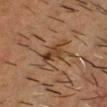biopsy status: imaged on a skin check; not biopsied | image source: ~15 mm crop, total-body skin-cancer survey | diameter: about 3 mm | site: the head or neck | image-analysis metrics: a lesion color around L≈32 a*≈16 b*≈27 in CIELAB, a lesion–skin lightness drop of about 8, and a lesion-to-skin contrast of about 8 (normalized; higher = more distinct); a border-irregularity rating of about 3.5/10 and radial color variation of about 2; an automated nevus-likeness rating near 50 out of 100 and a detector confidence of about 100 out of 100 that the crop contains a lesion | subject: male, in their 60s.